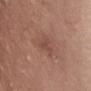lesion size: ≈3.5 mm
lighting: white-light illumination
acquisition: 15 mm crop, total-body photography
subject: female, about 65 years old
anatomic site: the abdomen
automated lesion analysis: border irregularity of about 5.5 on a 0–10 scale, internal color variation of about 1 on a 0–10 scale, and peripheral color asymmetry of about 0; an automated nevus-likeness rating near 0 out of 100